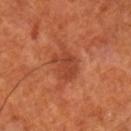No biopsy was performed on this lesion — it was imaged during a full skin examination and was not determined to be concerning. The lesion's longest dimension is about 4 mm. A close-up tile cropped from a whole-body skin photograph, about 15 mm across. A male subject, roughly 70 years of age. This is a cross-polarized tile. On the right lower leg.